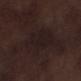Notes:
- notes: no biopsy performed (imaged during a skin exam)
- diameter: ≈6 mm
- subject: male, aged around 70
- imaging modality: 15 mm crop, total-body photography
- anatomic site: the left lower leg